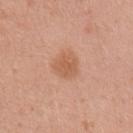Impression:
Imaged during a routine full-body skin examination; the lesion was not biopsied and no histopathology is available.
Acquisition and patient details:
A female patient, roughly 30 years of age. Imaged with white-light lighting. From the right upper arm. Measured at roughly 3 mm in maximum diameter. This image is a 15 mm lesion crop taken from a total-body photograph. An algorithmic analysis of the crop reported an automated nevus-likeness rating near 45 out of 100 and lesion-presence confidence of about 100/100.